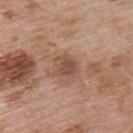Q: Was this lesion biopsied?
A: imaged on a skin check; not biopsied
Q: Lesion location?
A: the upper back
Q: Patient demographics?
A: male, aged around 55
Q: What kind of image is this?
A: 15 mm crop, total-body photography
Q: How large is the lesion?
A: ~3 mm (longest diameter)